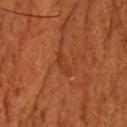notes=no biopsy performed (imaged during a skin exam)
image source=~15 mm tile from a whole-body skin photo
size=about 3 mm
anatomic site=the upper back
image-analysis metrics=a lesion area of about 3 mm², an eccentricity of roughly 0.95, and a symmetry-axis asymmetry near 0.45; a lesion color around L≈31 a*≈25 b*≈31 in CIELAB, a lesion–skin lightness drop of about 5, and a normalized border contrast of about 4.5; a classifier nevus-likeness of about 0/100 and a detector confidence of about 90 out of 100 that the crop contains a lesion
tile lighting=cross-polarized illumination
subject=male, roughly 60 years of age